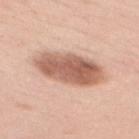Part of a total-body skin-imaging series; this lesion was reviewed on a skin check and was not flagged for biopsy. Located on the mid back. A region of skin cropped from a whole-body photographic capture, roughly 15 mm wide. A female subject, roughly 35 years of age.